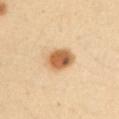| field | value |
|---|---|
| lesion size | about 4 mm |
| tile lighting | cross-polarized illumination |
| acquisition | total-body-photography crop, ~15 mm field of view |
| subject | female, in their mid- to late 30s |
| TBP lesion metrics | border irregularity of about 1.5 on a 0–10 scale, a within-lesion color-variation index near 7/10, and a peripheral color-asymmetry measure near 2.5; an automated nevus-likeness rating near 100 out of 100 and a lesion-detection confidence of about 100/100 |
| location | the arm |